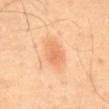Part of a total-body skin-imaging series; this lesion was reviewed on a skin check and was not flagged for biopsy. A 15 mm crop from a total-body photograph taken for skin-cancer surveillance. The lesion is located on the front of the torso. A male patient, aged approximately 65.